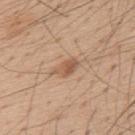Q: Was this lesion biopsied?
A: no biopsy performed (imaged during a skin exam)
Q: Automated lesion metrics?
A: a lesion color around L≈56 a*≈19 b*≈32 in CIELAB, roughly 10 lightness units darker than nearby skin, and a normalized border contrast of about 7; a border-irregularity index near 3/10 and internal color variation of about 4 on a 0–10 scale
Q: Where on the body is the lesion?
A: the upper back
Q: What is the imaging modality?
A: total-body-photography crop, ~15 mm field of view
Q: Who is the patient?
A: male, in their mid- to late 50s
Q: How large is the lesion?
A: ~3 mm (longest diameter)